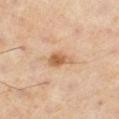| feature | finding |
|---|---|
| biopsy status | total-body-photography surveillance lesion; no biopsy |
| illumination | cross-polarized |
| anatomic site | the right thigh |
| image | ~15 mm crop, total-body skin-cancer survey |
| patient | male, about 55 years old |